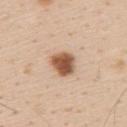Recorded during total-body skin imaging; not selected for excision or biopsy.
A male patient, aged 58–62.
On the upper back.
A lesion tile, about 15 mm wide, cut from a 3D total-body photograph.
Measured at roughly 3.5 mm in maximum diameter.
The tile uses white-light illumination.